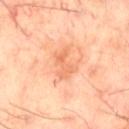Q: Was this lesion biopsied?
A: catalogued during a skin exam; not biopsied
Q: Illumination type?
A: cross-polarized illumination
Q: How was this image acquired?
A: total-body-photography crop, ~15 mm field of view
Q: Automated lesion metrics?
A: a mean CIELAB color near L≈65 a*≈27 b*≈38, roughly 8 lightness units darker than nearby skin, and a normalized border contrast of about 5.5; a border-irregularity rating of about 5.5/10, a color-variation rating of about 0.5/10, and radial color variation of about 0; an automated nevus-likeness rating near 0 out of 100 and lesion-presence confidence of about 100/100
Q: Where on the body is the lesion?
A: the leg
Q: Who is the patient?
A: male, roughly 60 years of age